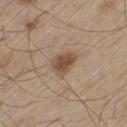Q: Is there a histopathology result?
A: total-body-photography surveillance lesion; no biopsy
Q: Lesion size?
A: ~3.5 mm (longest diameter)
Q: Lesion location?
A: the left thigh
Q: Who is the patient?
A: male, aged 58 to 62
Q: Automated lesion metrics?
A: a lesion area of about 6.5 mm² and two-axis asymmetry of about 0.3; a lesion color around L≈49 a*≈16 b*≈28 in CIELAB, a lesion–skin lightness drop of about 11, and a normalized lesion–skin contrast near 8.5; an automated nevus-likeness rating near 90 out of 100
Q: How was this image acquired?
A: total-body-photography crop, ~15 mm field of view
Q: What lighting was used for the tile?
A: white-light illumination